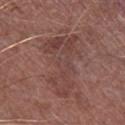This is a white-light tile.
An algorithmic analysis of the crop reported a lesion area of about 20 mm². The analysis additionally found roughly 6 lightness units darker than nearby skin and a normalized border contrast of about 5.5. The analysis additionally found a classifier nevus-likeness of about 0/100 and lesion-presence confidence of about 75/100.
The subject is a male aged around 75.
A region of skin cropped from a whole-body photographic capture, roughly 15 mm wide.
The lesion is located on the right lower leg.